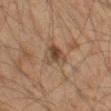biopsy status: catalogued during a skin exam; not biopsied | lighting: cross-polarized | acquisition: total-body-photography crop, ~15 mm field of view | automated lesion analysis: a lesion area of about 5.5 mm², a shape eccentricity near 0.35, and a symmetry-axis asymmetry near 0.4; an average lesion color of about L≈37 a*≈13 b*≈25 (CIELAB) and a lesion-to-skin contrast of about 8 (normalized; higher = more distinct) | lesion size: about 2.5 mm | anatomic site: the left thigh | subject: male, roughly 60 years of age.